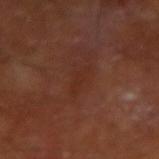• biopsy status: total-body-photography surveillance lesion; no biopsy
• site: the right forearm
• imaging modality: ~15 mm crop, total-body skin-cancer survey
• automated lesion analysis: a mean CIELAB color near L≈27 a*≈22 b*≈25, roughly 4 lightness units darker than nearby skin, and a normalized lesion–skin contrast near 4.5; a border-irregularity index near 5/10, a within-lesion color-variation index near 0/10, and peripheral color asymmetry of about 0
• subject: male, aged 63–67